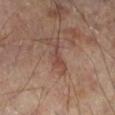Clinical impression:
Imaged during a routine full-body skin examination; the lesion was not biopsied and no histopathology is available.
Clinical summary:
From the left lower leg. Automated image analysis of the tile measured a lesion–skin lightness drop of about 7. The software also gave a nevus-likeness score of about 0/100. A male patient roughly 65 years of age. Imaged with cross-polarized lighting. Approximately 3 mm at its widest. A 15 mm crop from a total-body photograph taken for skin-cancer surveillance.